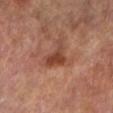| feature | finding |
|---|---|
| lighting | cross-polarized |
| subject | female, aged 73–77 |
| image | 15 mm crop, total-body photography |
| site | the right lower leg |
| automated metrics | an eccentricity of roughly 0.7 and a symmetry-axis asymmetry near 0.55; a lesion color around L≈43 a*≈25 b*≈30 in CIELAB, about 10 CIELAB-L* units darker than the surrounding skin, and a normalized border contrast of about 8; a nevus-likeness score of about 0/100 and lesion-presence confidence of about 100/100 |
| lesion size | ~3.5 mm (longest diameter) |